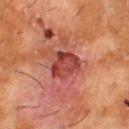<record>
  <biopsy_status>not biopsied; imaged during a skin examination</biopsy_status>
  <automated_metrics>
    <area_mm2_approx>9.5</area_mm2_approx>
    <shape_asymmetry>0.25</shape_asymmetry>
    <vs_skin_darker_L>11.0</vs_skin_darker_L>
    <vs_skin_contrast_norm>8.5</vs_skin_contrast_norm>
    <border_irregularity_0_10>2.5</border_irregularity_0_10>
    <color_variation_0_10>5.5</color_variation_0_10>
    <peripheral_color_asymmetry>1.5</peripheral_color_asymmetry>
    <nevus_likeness_0_100>0</nevus_likeness_0_100>
    <lesion_detection_confidence_0_100>100</lesion_detection_confidence_0_100>
  </automated_metrics>
  <lighting>cross-polarized</lighting>
  <image>
    <source>total-body photography crop</source>
    <field_of_view_mm>15</field_of_view_mm>
  </image>
  <lesion_size>
    <long_diameter_mm_approx>3.5</long_diameter_mm_approx>
  </lesion_size>
  <patient>
    <sex>male</sex>
    <age_approx>60</age_approx>
  </patient>
  <site>leg</site>
</record>